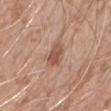Case summary:
* workup · total-body-photography surveillance lesion; no biopsy
* patient · male, in their 60s
* imaging modality · total-body-photography crop, ~15 mm field of view
* location · the left forearm